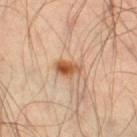The lesion was photographed on a routine skin check and not biopsied; there is no pathology result. Located on the leg. Longest diameter approximately 3 mm. The lesion-visualizer software estimated a nevus-likeness score of about 100/100 and a detector confidence of about 100 out of 100 that the crop contains a lesion. A male subject aged 53–57. A 15 mm close-up tile from a total-body photography series done for melanoma screening. The tile uses cross-polarized illumination.